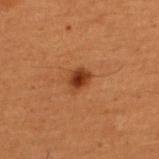notes = imaged on a skin check; not biopsied
anatomic site = the back
lighting = cross-polarized
image source = ~15 mm crop, total-body skin-cancer survey
automated metrics = a footprint of about 4.5 mm², an eccentricity of roughly 0.6, and a symmetry-axis asymmetry near 0.2; a color-variation rating of about 2.5/10; an automated nevus-likeness rating near 95 out of 100 and a lesion-detection confidence of about 100/100
patient = male, roughly 50 years of age
diameter = about 2.5 mm